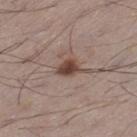Impression: Recorded during total-body skin imaging; not selected for excision or biopsy. Background: The lesion-visualizer software estimated a footprint of about 4.5 mm², an outline eccentricity of about 0.7 (0 = round, 1 = elongated), and a symmetry-axis asymmetry near 0.3. And it measured a mean CIELAB color near L≈44 a*≈17 b*≈24, a lesion–skin lightness drop of about 13, and a lesion-to-skin contrast of about 10.5 (normalized; higher = more distinct). From the left thigh. A male patient aged 48 to 52. Captured under white-light illumination. Cropped from a total-body skin-imaging series; the visible field is about 15 mm. Measured at roughly 3 mm in maximum diameter.